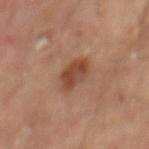Clinical impression: This lesion was catalogued during total-body skin photography and was not selected for biopsy. Clinical summary: Measured at roughly 3.5 mm in maximum diameter. A 15 mm close-up tile from a total-body photography series done for melanoma screening. A male subject, about 65 years old. From the mid back. An algorithmic analysis of the crop reported a classifier nevus-likeness of about 80/100 and lesion-presence confidence of about 100/100.